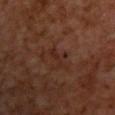| field | value |
|---|---|
| lighting | cross-polarized illumination |
| image source | total-body-photography crop, ~15 mm field of view |
| automated lesion analysis | a footprint of about 4 mm² and a shape-asymmetry score of about 0.5 (0 = symmetric) |
| lesion diameter | about 2.5 mm |
| subject | male, aged approximately 70 |
| body site | the chest |